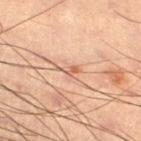<case>
<lesion_size>
  <long_diameter_mm_approx>3.0</long_diameter_mm_approx>
</lesion_size>
<site>right thigh</site>
<patient>
  <sex>male</sex>
  <age_approx>55</age_approx>
</patient>
<image>
  <source>total-body photography crop</source>
  <field_of_view_mm>15</field_of_view_mm>
</image>
<lighting>cross-polarized</lighting>
<automated_metrics>
  <nevus_likeness_0_100>0</nevus_likeness_0_100>
  <lesion_detection_confidence_0_100>80</lesion_detection_confidence_0_100>
</automated_metrics>
</case>